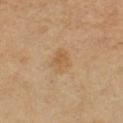Findings:
* notes: catalogued during a skin exam; not biopsied
* patient: female, aged 48–52
* body site: the left forearm
* imaging modality: ~15 mm crop, total-body skin-cancer survey